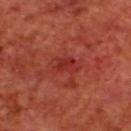Q: Is there a histopathology result?
A: imaged on a skin check; not biopsied
Q: What is the lesion's diameter?
A: ≈3 mm
Q: Where on the body is the lesion?
A: the upper back
Q: How was this image acquired?
A: 15 mm crop, total-body photography
Q: What did automated image analysis measure?
A: a lesion area of about 4 mm², a shape eccentricity near 0.8, and a symmetry-axis asymmetry near 0.4; a border-irregularity rating of about 5/10, a within-lesion color-variation index near 3/10, and peripheral color asymmetry of about 1.5; a detector confidence of about 95 out of 100 that the crop contains a lesion
Q: Patient demographics?
A: male, in their 70s